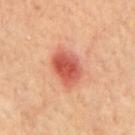biopsy status=imaged on a skin check; not biopsied
subject=male, aged 68–72
tile lighting=cross-polarized
body site=the mid back
diameter=≈4 mm
image-analysis metrics=an automated nevus-likeness rating near 100 out of 100 and lesion-presence confidence of about 100/100
imaging modality=total-body-photography crop, ~15 mm field of view